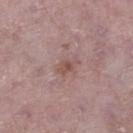follow-up=catalogued during a skin exam; not biopsied | tile lighting=white-light | lesion diameter=≈2.5 mm | image-analysis metrics=a lesion color around L≈51 a*≈20 b*≈21 in CIELAB and a lesion–skin lightness drop of about 8; a border-irregularity index near 4.5/10 and radial color variation of about 0.5; a nevus-likeness score of about 0/100 and a lesion-detection confidence of about 100/100 | image=total-body-photography crop, ~15 mm field of view | anatomic site=the right lower leg | subject=male, aged around 75.